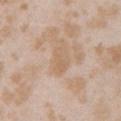The lesion was tiled from a total-body skin photograph and was not biopsied. Captured under white-light illumination. On the left upper arm. Automated tile analysis of the lesion measured a shape-asymmetry score of about 0.3 (0 = symmetric). A female subject, about 25 years old. The recorded lesion diameter is about 3.5 mm. A close-up tile cropped from a whole-body skin photograph, about 15 mm across.